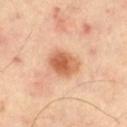Assessment:
The lesion was tiled from a total-body skin photograph and was not biopsied.
Context:
Imaged with cross-polarized lighting. The lesion is located on the left thigh. Cropped from a whole-body photographic skin survey; the tile spans about 15 mm. Measured at roughly 3.5 mm in maximum diameter. An algorithmic analysis of the crop reported a border-irregularity index near 1.5/10, a color-variation rating of about 5.5/10, and radial color variation of about 1.5. And it measured an automated nevus-likeness rating near 100 out of 100 and lesion-presence confidence of about 100/100.